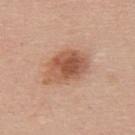biopsy status: catalogued during a skin exam; not biopsied
subject: female, in their 40s
size: about 6 mm
site: the upper back
imaging modality: ~15 mm crop, total-body skin-cancer survey
automated metrics: a lesion area of about 16 mm² and an eccentricity of roughly 0.8; an average lesion color of about L≈55 a*≈23 b*≈32 (CIELAB), a lesion–skin lightness drop of about 12, and a lesion-to-skin contrast of about 8.5 (normalized; higher = more distinct); a border-irregularity rating of about 2.5/10, a color-variation rating of about 6/10, and peripheral color asymmetry of about 2